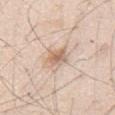Q: Was this lesion biopsied?
A: no biopsy performed (imaged during a skin exam)
Q: What is the imaging modality?
A: 15 mm crop, total-body photography
Q: What lighting was used for the tile?
A: white-light
Q: Who is the patient?
A: male, aged approximately 45
Q: Where on the body is the lesion?
A: the chest